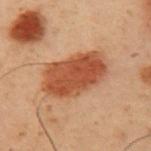A male subject, aged 53–57. Measured at roughly 6.5 mm in maximum diameter. Cropped from a total-body skin-imaging series; the visible field is about 15 mm. This is a cross-polarized tile. The lesion is located on the arm. Automated tile analysis of the lesion measured a classifier nevus-likeness of about 100/100 and a detector confidence of about 100 out of 100 that the crop contains a lesion.